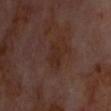notes = imaged on a skin check; not biopsied